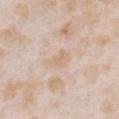Assessment:
Captured during whole-body skin photography for melanoma surveillance; the lesion was not biopsied.
Acquisition and patient details:
The tile uses white-light illumination. On the front of the torso. Measured at roughly 3 mm in maximum diameter. Cropped from a whole-body photographic skin survey; the tile spans about 15 mm. The patient is a female aged approximately 25. Automated tile analysis of the lesion measured a lesion color around L≈69 a*≈14 b*≈30 in CIELAB, roughly 6 lightness units darker than nearby skin, and a normalized lesion–skin contrast near 5. It also reported border irregularity of about 3.5 on a 0–10 scale, internal color variation of about 1.5 on a 0–10 scale, and a peripheral color-asymmetry measure near 0.5. And it measured an automated nevus-likeness rating near 0 out of 100.